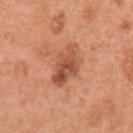Q: Was this lesion biopsied?
A: total-body-photography surveillance lesion; no biopsy
Q: How was this image acquired?
A: ~15 mm crop, total-body skin-cancer survey
Q: Patient demographics?
A: female, approximately 40 years of age
Q: Where on the body is the lesion?
A: the left upper arm